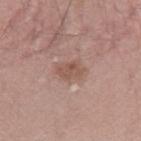Part of a total-body skin-imaging series; this lesion was reviewed on a skin check and was not flagged for biopsy. Longest diameter approximately 3.5 mm. A male subject, aged 58 to 62. The tile uses white-light illumination. The lesion is on the arm. A region of skin cropped from a whole-body photographic capture, roughly 15 mm wide.